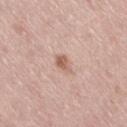This lesion was catalogued during total-body skin photography and was not selected for biopsy. The tile uses white-light illumination. A roughly 15 mm field-of-view crop from a total-body skin photograph. Automated image analysis of the tile measured a lesion area of about 3.5 mm², an outline eccentricity of about 0.8 (0 = round, 1 = elongated), and a symmetry-axis asymmetry near 0.25. It also reported a lesion color around L≈60 a*≈21 b*≈27 in CIELAB, roughly 11 lightness units darker than nearby skin, and a normalized lesion–skin contrast near 7. The software also gave border irregularity of about 2 on a 0–10 scale. The analysis additionally found an automated nevus-likeness rating near 60 out of 100 and lesion-presence confidence of about 100/100. A female patient, aged 63 to 67. From the right thigh. Longest diameter approximately 2.5 mm.